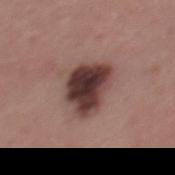Q: Was this lesion biopsied?
A: imaged on a skin check; not biopsied
Q: What kind of image is this?
A: ~15 mm tile from a whole-body skin photo
Q: What did automated image analysis measure?
A: a lesion area of about 15 mm², an eccentricity of roughly 0.65, and a shape-asymmetry score of about 0.3 (0 = symmetric); an automated nevus-likeness rating near 55 out of 100
Q: How was the tile lit?
A: white-light illumination
Q: What is the anatomic site?
A: the mid back
Q: Lesion size?
A: about 5 mm
Q: Patient demographics?
A: male, roughly 40 years of age